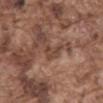The lesion was tiled from a total-body skin photograph and was not biopsied. The lesion-visualizer software estimated a lesion color around L≈43 a*≈19 b*≈26 in CIELAB, about 9 CIELAB-L* units darker than the surrounding skin, and a lesion-to-skin contrast of about 7 (normalized; higher = more distinct). The software also gave a border-irregularity rating of about 5.5/10, internal color variation of about 1 on a 0–10 scale, and radial color variation of about 0. Located on the abdomen. Approximately 2.5 mm at its widest. A male subject, about 75 years old. A lesion tile, about 15 mm wide, cut from a 3D total-body photograph. This is a white-light tile.